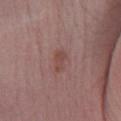A close-up tile cropped from a whole-body skin photograph, about 15 mm across. Located on the right lower leg. A female patient, in their mid- to late 40s. Measured at roughly 2.5 mm in maximum diameter.